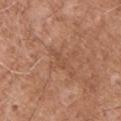<record>
<biopsy_status>not biopsied; imaged during a skin examination</biopsy_status>
<lighting>white-light</lighting>
<lesion_size>
  <long_diameter_mm_approx>3.0</long_diameter_mm_approx>
</lesion_size>
<image>
  <source>total-body photography crop</source>
  <field_of_view_mm>15</field_of_view_mm>
</image>
<automated_metrics>
  <eccentricity>0.95</eccentricity>
  <shape_asymmetry>0.45</shape_asymmetry>
  <vs_skin_contrast_norm>4.5</vs_skin_contrast_norm>
  <border_irregularity_0_10>5.5</border_irregularity_0_10>
  <color_variation_0_10>0.0</color_variation_0_10>
  <peripheral_color_asymmetry>0.0</peripheral_color_asymmetry>
  <nevus_likeness_0_100>0</nevus_likeness_0_100>
  <lesion_detection_confidence_0_100>95</lesion_detection_confidence_0_100>
</automated_metrics>
<patient>
  <sex>male</sex>
  <age_approx>55</age_approx>
</patient>
<site>back</site>
</record>